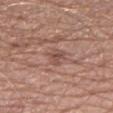Captured during whole-body skin photography for melanoma surveillance; the lesion was not biopsied. The lesion is located on the left forearm. About 2.5 mm across. Cropped from a whole-body photographic skin survey; the tile spans about 15 mm. The subject is a male aged 63 to 67.